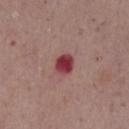No biopsy was performed on this lesion — it was imaged during a full skin examination and was not determined to be concerning. The lesion is located on the chest. The total-body-photography lesion software estimated a footprint of about 5 mm², an outline eccentricity of about 0.55 (0 = round, 1 = elongated), and two-axis asymmetry of about 0.15. It also reported border irregularity of about 1 on a 0–10 scale and a peripheral color-asymmetry measure near 1. The software also gave an automated nevus-likeness rating near 0 out of 100 and a detector confidence of about 100 out of 100 that the crop contains a lesion. A region of skin cropped from a whole-body photographic capture, roughly 15 mm wide. Approximately 3 mm at its widest. A male patient, aged approximately 70.